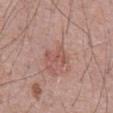  biopsy_status: not biopsied; imaged during a skin examination
  site: chest
  lesion_size:
    long_diameter_mm_approx: 3.0
  patient:
    sex: male
    age_approx: 70
  image:
    source: total-body photography crop
    field_of_view_mm: 15